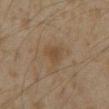Part of a total-body skin-imaging series; this lesion was reviewed on a skin check and was not flagged for biopsy. This is a cross-polarized tile. The lesion-visualizer software estimated an average lesion color of about L≈41 a*≈13 b*≈28 (CIELAB), about 5 CIELAB-L* units darker than the surrounding skin, and a lesion-to-skin contrast of about 5.5 (normalized; higher = more distinct). It also reported a border-irregularity index near 3.5/10, a color-variation rating of about 1.5/10, and radial color variation of about 0.5. The analysis additionally found a nevus-likeness score of about 0/100 and a detector confidence of about 100 out of 100 that the crop contains a lesion. Located on the front of the torso. Measured at roughly 2.5 mm in maximum diameter. A male subject, aged approximately 60. A 15 mm crop from a total-body photograph taken for skin-cancer surveillance.